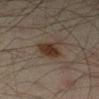biopsy_status: not biopsied; imaged during a skin examination
image:
  source: total-body photography crop
  field_of_view_mm: 15
patient:
  sex: male
  age_approx: 45
site: left lower leg
lesion_size:
  long_diameter_mm_approx: 3.5
lighting: cross-polarized